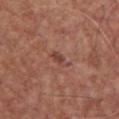Part of a total-body skin-imaging series; this lesion was reviewed on a skin check and was not flagged for biopsy. A male patient aged 63 to 67. A 15 mm close-up extracted from a 3D total-body photography capture. This is a white-light tile. From the chest. An algorithmic analysis of the crop reported border irregularity of about 4 on a 0–10 scale and a within-lesion color-variation index near 0.5/10. Measured at roughly 3 mm in maximum diameter.